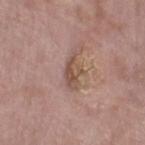Captured during whole-body skin photography for melanoma surveillance; the lesion was not biopsied. The patient is a female aged approximately 70. Approximately 4 mm at its widest. Located on the right lower leg. A lesion tile, about 15 mm wide, cut from a 3D total-body photograph. Automated image analysis of the tile measured about 9 CIELAB-L* units darker than the surrounding skin. The software also gave a border-irregularity index near 5/10, internal color variation of about 4 on a 0–10 scale, and radial color variation of about 1.5.